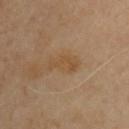notes=no biopsy performed (imaged during a skin exam); imaging modality=total-body-photography crop, ~15 mm field of view; anatomic site=the chest; lesion size=~3.5 mm (longest diameter); illumination=cross-polarized; patient=male, approximately 70 years of age.